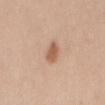Findings:
- follow-up: total-body-photography surveillance lesion; no biopsy
- subject: female, roughly 25 years of age
- lesion diameter: ~3 mm (longest diameter)
- location: the mid back
- imaging modality: total-body-photography crop, ~15 mm field of view